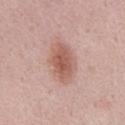biopsy status: total-body-photography surveillance lesion; no biopsy
size: ~4.5 mm (longest diameter)
image-analysis metrics: a classifier nevus-likeness of about 95/100 and lesion-presence confidence of about 100/100
location: the abdomen
image: ~15 mm crop, total-body skin-cancer survey
lighting: white-light
patient: male, aged 58 to 62